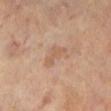Captured during whole-body skin photography for melanoma surveillance; the lesion was not biopsied. Longest diameter approximately 3.5 mm. The tile uses cross-polarized illumination. A region of skin cropped from a whole-body photographic capture, roughly 15 mm wide. The lesion-visualizer software estimated an area of roughly 5 mm². And it measured an average lesion color of about L≈56 a*≈18 b*≈30 (CIELAB), roughly 6 lightness units darker than nearby skin, and a normalized lesion–skin contrast near 4.5. It also reported border irregularity of about 5 on a 0–10 scale, a color-variation rating of about 1/10, and peripheral color asymmetry of about 0.5. The patient is a female aged 63–67. Located on the right leg.